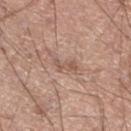Image and clinical context: On the leg. Captured under white-light illumination. About 3 mm across. A male patient, aged approximately 20. A lesion tile, about 15 mm wide, cut from a 3D total-body photograph.